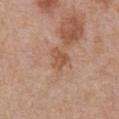Assessment:
Captured during whole-body skin photography for melanoma surveillance; the lesion was not biopsied.
Context:
A 15 mm close-up extracted from a 3D total-body photography capture. A male patient, aged 68 to 72. Located on the front of the torso. An algorithmic analysis of the crop reported a footprint of about 4.5 mm², a shape eccentricity near 0.5, and two-axis asymmetry of about 0.4. The analysis additionally found an average lesion color of about L≈53 a*≈21 b*≈32 (CIELAB). And it measured a border-irregularity index near 4/10, a color-variation rating of about 2.5/10, and peripheral color asymmetry of about 1. The lesion's longest dimension is about 2.5 mm.